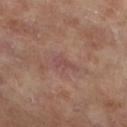The lesion is located on the right lower leg.
The tile uses cross-polarized illumination.
Cropped from a whole-body photographic skin survey; the tile spans about 15 mm.
Longest diameter approximately 3 mm.
A female patient roughly 75 years of age.